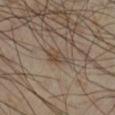<tbp_lesion>
  <lighting>cross-polarized</lighting>
  <image>
    <source>total-body photography crop</source>
    <field_of_view_mm>15</field_of_view_mm>
  </image>
  <lesion_size>
    <long_diameter_mm_approx>2.5</long_diameter_mm_approx>
  </lesion_size>
  <site>right lower leg</site>
  <patient>
    <sex>male</sex>
    <age_approx>40</age_approx>
  </patient>
</tbp_lesion>